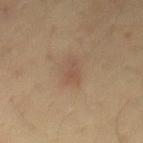{"biopsy_status": "not biopsied; imaged during a skin examination", "image": {"source": "total-body photography crop", "field_of_view_mm": 15}, "site": "mid back", "patient": {"sex": "male", "age_approx": 35}}